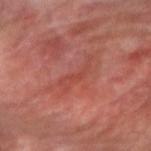  biopsy_status: not biopsied; imaged during a skin examination
  lighting: cross-polarized
  site: right forearm
  image:
    source: total-body photography crop
    field_of_view_mm: 15
  patient:
    sex: male
    age_approx: 70